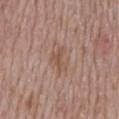Findings:
- workup: catalogued during a skin exam; not biopsied
- lesion size: ~3.5 mm (longest diameter)
- anatomic site: the back
- automated metrics: a lesion area of about 4.5 mm² and an eccentricity of roughly 0.85; a within-lesion color-variation index near 2/10 and a peripheral color-asymmetry measure near 0.5; a nevus-likeness score of about 0/100 and a lesion-detection confidence of about 100/100
- image: total-body-photography crop, ~15 mm field of view
- lighting: white-light illumination
- patient: male, aged around 75